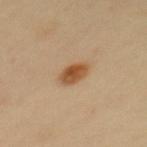The subject is a female in their 40s. This is a cross-polarized tile. The lesion's longest dimension is about 3 mm. Located on the upper back. A roughly 15 mm field-of-view crop from a total-body skin photograph.